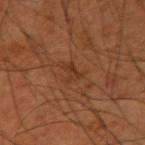Clinical impression:
The lesion was tiled from a total-body skin photograph and was not biopsied.
Image and clinical context:
The tile uses cross-polarized illumination. Cropped from a total-body skin-imaging series; the visible field is about 15 mm. The lesion-visualizer software estimated an area of roughly 4 mm². The lesion is on the right upper arm. A male patient, about 60 years old.